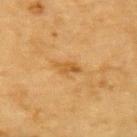Assessment: Captured during whole-body skin photography for melanoma surveillance; the lesion was not biopsied. Clinical summary: A male patient in their mid-80s or older. Approximately 3 mm at its widest. Captured under cross-polarized illumination. Located on the upper back. A lesion tile, about 15 mm wide, cut from a 3D total-body photograph.